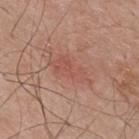biopsy status=catalogued during a skin exam; not biopsied
patient=male, aged approximately 70
automated metrics=a lesion area of about 9 mm², an eccentricity of roughly 0.9, and a symmetry-axis asymmetry near 0.3; a mean CIELAB color near L≈53 a*≈25 b*≈28, roughly 6 lightness units darker than nearby skin, and a normalized border contrast of about 4.5
image=total-body-photography crop, ~15 mm field of view
body site=the upper back
lighting=white-light
lesion diameter=~5.5 mm (longest diameter)